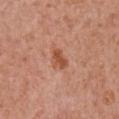location: the chest
diameter: about 3 mm
automated lesion analysis: an average lesion color of about L≈52 a*≈26 b*≈34 (CIELAB), roughly 10 lightness units darker than nearby skin, and a normalized lesion–skin contrast near 7.5; a color-variation rating of about 2/10
acquisition: ~15 mm crop, total-body skin-cancer survey
patient: female, approximately 70 years of age
illumination: white-light